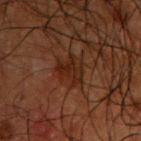Q: Is there a histopathology result?
A: total-body-photography surveillance lesion; no biopsy
Q: Where on the body is the lesion?
A: the upper back
Q: Illumination type?
A: cross-polarized illumination
Q: What is the lesion's diameter?
A: ≈3.5 mm
Q: What are the patient's age and sex?
A: male, aged around 50
Q: What is the imaging modality?
A: ~15 mm crop, total-body skin-cancer survey